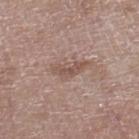{
  "biopsy_status": "not biopsied; imaged during a skin examination",
  "lighting": "white-light",
  "patient": {
    "sex": "male",
    "age_approx": 80
  },
  "site": "right lower leg",
  "lesion_size": {
    "long_diameter_mm_approx": 4.0
  },
  "image": {
    "source": "total-body photography crop",
    "field_of_view_mm": 15
  }
}